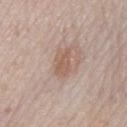{
  "biopsy_status": "not biopsied; imaged during a skin examination",
  "patient": {
    "sex": "female",
    "age_approx": 60
  },
  "image": {
    "source": "total-body photography crop",
    "field_of_view_mm": 15
  },
  "site": "left lower leg",
  "lighting": "white-light",
  "lesion_size": {
    "long_diameter_mm_approx": 3.5
  }
}